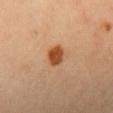Q: Was a biopsy performed?
A: total-body-photography surveillance lesion; no biopsy
Q: What kind of image is this?
A: 15 mm crop, total-body photography
Q: Patient demographics?
A: female, aged approximately 35
Q: What is the anatomic site?
A: the head or neck
Q: How large is the lesion?
A: ≈2.5 mm
Q: Automated lesion metrics?
A: an average lesion color of about L≈48 a*≈26 b*≈38 (CIELAB), roughly 14 lightness units darker than nearby skin, and a normalized border contrast of about 11
Q: How was the tile lit?
A: cross-polarized illumination